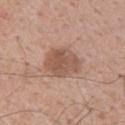The subject is a male aged approximately 65.
Automated tile analysis of the lesion measured a mean CIELAB color near L≈54 a*≈20 b*≈28, a lesion–skin lightness drop of about 11, and a lesion-to-skin contrast of about 7.5 (normalized; higher = more distinct). The software also gave an automated nevus-likeness rating near 10 out of 100 and a lesion-detection confidence of about 100/100.
The lesion is located on the upper back.
A 15 mm close-up extracted from a 3D total-body photography capture.
Longest diameter approximately 4.5 mm.
Imaged with white-light lighting.